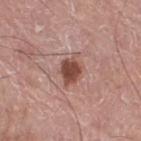follow-up: total-body-photography surveillance lesion; no biopsy
body site: the leg
illumination: white-light illumination
diameter: ≈4 mm
subject: male, aged around 75
acquisition: 15 mm crop, total-body photography